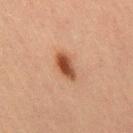Captured during whole-body skin photography for melanoma surveillance; the lesion was not biopsied. A male patient roughly 70 years of age. Cropped from a whole-body photographic skin survey; the tile spans about 15 mm. The recorded lesion diameter is about 3.5 mm. Located on the leg. Captured under cross-polarized illumination.